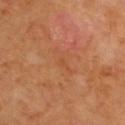Q: Is there a histopathology result?
A: catalogued during a skin exam; not biopsied
Q: What is the imaging modality?
A: ~15 mm tile from a whole-body skin photo
Q: Lesion location?
A: the upper back
Q: What are the patient's age and sex?
A: approximately 70 years of age
Q: What is the lesion's diameter?
A: about 1 mm
Q: How was the tile lit?
A: cross-polarized
Q: What did automated image analysis measure?
A: a footprint of about 1 mm², an eccentricity of roughly 0.8, and two-axis asymmetry of about 0.25; border irregularity of about 1.5 on a 0–10 scale and radial color variation of about 0